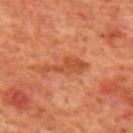Assessment: This lesion was catalogued during total-body skin photography and was not selected for biopsy. Acquisition and patient details: Captured under cross-polarized illumination. An algorithmic analysis of the crop reported a lesion area of about 7.5 mm², an outline eccentricity of about 0.95 (0 = round, 1 = elongated), and a symmetry-axis asymmetry near 0.5. The software also gave a lesion-to-skin contrast of about 6 (normalized; higher = more distinct). A male patient, in their 70s. Longest diameter approximately 5.5 mm. A lesion tile, about 15 mm wide, cut from a 3D total-body photograph. Located on the mid back.